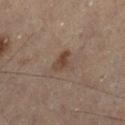Notes:
* notes — no biopsy performed (imaged during a skin exam)
* location — the leg
* patient — male, aged 53 to 57
* image source — 15 mm crop, total-body photography
* size — ~2.5 mm (longest diameter)
* lighting — cross-polarized illumination